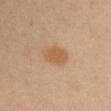Captured during whole-body skin photography for melanoma surveillance; the lesion was not biopsied. Automated image analysis of the tile measured a border-irregularity index near 2/10, a color-variation rating of about 1.5/10, and peripheral color asymmetry of about 0.5. It also reported an automated nevus-likeness rating near 90 out of 100 and a detector confidence of about 100 out of 100 that the crop contains a lesion. A lesion tile, about 15 mm wide, cut from a 3D total-body photograph. Imaged with cross-polarized lighting. The lesion is on the left upper arm. A female patient, about 40 years old. The recorded lesion diameter is about 3.5 mm.